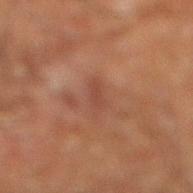{"automated_metrics": {"area_mm2_approx": 3.5, "eccentricity": 0.9, "shape_asymmetry": 0.25, "cielab_L": 37, "cielab_a": 20, "cielab_b": 25, "vs_skin_darker_L": 5.0, "vs_skin_contrast_norm": 5.0}, "lesion_size": {"long_diameter_mm_approx": 3.0}, "image": {"source": "total-body photography crop", "field_of_view_mm": 15}, "site": "right lower leg", "patient": {"sex": "male", "age_approx": 65}}